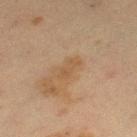Q: What kind of image is this?
A: ~15 mm crop, total-body skin-cancer survey
Q: Lesion location?
A: the left thigh
Q: Automated lesion metrics?
A: a mean CIELAB color near L≈43 a*≈15 b*≈30, roughly 5 lightness units darker than nearby skin, and a normalized lesion–skin contrast near 5.5; a classifier nevus-likeness of about 0/100 and a detector confidence of about 100 out of 100 that the crop contains a lesion
Q: Illumination type?
A: cross-polarized
Q: Patient demographics?
A: female, aged 38–42
Q: What is the lesion's diameter?
A: ~3 mm (longest diameter)